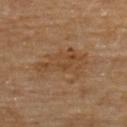biopsy status: total-body-photography surveillance lesion; no biopsy
lesion diameter: ~6.5 mm (longest diameter)
image: ~15 mm crop, total-body skin-cancer survey
body site: the back
subject: male, aged around 85 or older
image-analysis metrics: a footprint of about 15 mm² and an outline eccentricity of about 0.9 (0 = round, 1 = elongated); a classifier nevus-likeness of about 0/100 and lesion-presence confidence of about 100/100